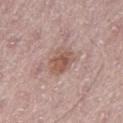notes = catalogued during a skin exam; not biopsied | patient = male, aged around 65 | body site = the left thigh | size = ~3.5 mm (longest diameter) | lighting = white-light illumination | acquisition = total-body-photography crop, ~15 mm field of view.